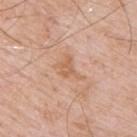  lighting: white-light
  image:
    source: total-body photography crop
    field_of_view_mm: 15
  patient:
    sex: male
    age_approx: 65
  lesion_size:
    long_diameter_mm_approx: 3.5
  site: upper back